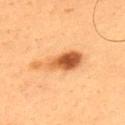Clinical summary:
The tile uses cross-polarized illumination. The lesion is on the upper back. The lesion-visualizer software estimated a lesion area of about 11 mm², an eccentricity of roughly 0.9, and a symmetry-axis asymmetry near 0.4. The software also gave lesion-presence confidence of about 100/100. The recorded lesion diameter is about 6 mm. The subject is a male aged around 55. A roughly 15 mm field-of-view crop from a total-body skin photograph.